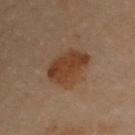notes: total-body-photography surveillance lesion; no biopsy | body site: the back | subject: female, aged 58–62 | lighting: cross-polarized illumination | lesion diameter: ~4.5 mm (longest diameter) | acquisition: total-body-photography crop, ~15 mm field of view.